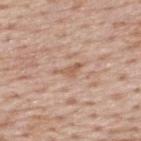workup — no biopsy performed (imaged during a skin exam) | lighting — white-light | lesion diameter — about 3 mm | patient — male, aged 73 to 77 | acquisition — 15 mm crop, total-body photography | site — the upper back | automated metrics — a color-variation rating of about 1/10 and peripheral color asymmetry of about 0.5; a classifier nevus-likeness of about 0/100 and a lesion-detection confidence of about 80/100.